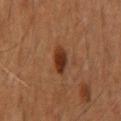Captured during whole-body skin photography for melanoma surveillance; the lesion was not biopsied. An algorithmic analysis of the crop reported an average lesion color of about L≈30 a*≈22 b*≈29 (CIELAB) and a lesion-to-skin contrast of about 10.5 (normalized; higher = more distinct). And it measured a border-irregularity rating of about 2/10, a within-lesion color-variation index near 3/10, and peripheral color asymmetry of about 1. A male patient, aged around 60. A lesion tile, about 15 mm wide, cut from a 3D total-body photograph. Imaged with cross-polarized lighting. On the abdomen. Approximately 3.5 mm at its widest.